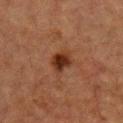Clinical impression: Part of a total-body skin-imaging series; this lesion was reviewed on a skin check and was not flagged for biopsy. Clinical summary: On the chest. Captured under cross-polarized illumination. Measured at roughly 2.5 mm in maximum diameter. A 15 mm close-up tile from a total-body photography series done for melanoma screening. A male patient aged 48 to 52.